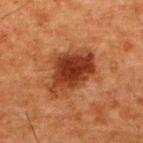The lesion was photographed on a routine skin check and not biopsied; there is no pathology result. The subject is a male aged 58–62. This is a cross-polarized tile. A 15 mm close-up extracted from a 3D total-body photography capture. The total-body-photography lesion software estimated a border-irregularity index near 3/10, internal color variation of about 5 on a 0–10 scale, and a peripheral color-asymmetry measure near 1.5. It also reported a classifier nevus-likeness of about 90/100. About 6 mm across. The lesion is located on the upper back.